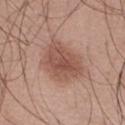{"biopsy_status": "not biopsied; imaged during a skin examination"}